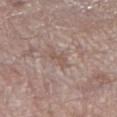Q: Is there a histopathology result?
A: catalogued during a skin exam; not biopsied
Q: What are the patient's age and sex?
A: male, approximately 70 years of age
Q: What is the imaging modality?
A: total-body-photography crop, ~15 mm field of view
Q: What is the anatomic site?
A: the leg